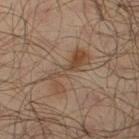subject — male, aged 63–67 | image source — total-body-photography crop, ~15 mm field of view | anatomic site — the right thigh.